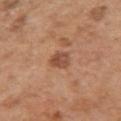The lesion was tiled from a total-body skin photograph and was not biopsied. This is a white-light tile. The total-body-photography lesion software estimated a mean CIELAB color near L≈49 a*≈23 b*≈31, a lesion–skin lightness drop of about 11, and a normalized lesion–skin contrast near 7.5. The lesion's longest dimension is about 2.5 mm. Cropped from a whole-body photographic skin survey; the tile spans about 15 mm. From the left upper arm. A male subject, in their mid-60s.